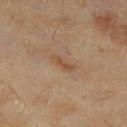{
  "biopsy_status": "not biopsied; imaged during a skin examination",
  "patient": {
    "sex": "female",
    "age_approx": 60
  },
  "site": "right lower leg",
  "automated_metrics": {
    "border_irregularity_0_10": 4.0,
    "color_variation_0_10": 0.0,
    "peripheral_color_asymmetry": 0.0,
    "nevus_likeness_0_100": 0,
    "lesion_detection_confidence_0_100": 100
  },
  "lighting": "cross-polarized",
  "lesion_size": {
    "long_diameter_mm_approx": 3.0
  },
  "image": {
    "source": "total-body photography crop",
    "field_of_view_mm": 15
  }
}